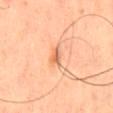Impression: Captured during whole-body skin photography for melanoma surveillance; the lesion was not biopsied. Context: The lesion is located on the mid back. A close-up tile cropped from a whole-body skin photograph, about 15 mm across. Captured under cross-polarized illumination. The patient is a male aged approximately 65. Longest diameter approximately 2.5 mm.